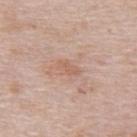<record>
<patient>
  <sex>female</sex>
  <age_approx>65</age_approx>
</patient>
<image>
  <source>total-body photography crop</source>
  <field_of_view_mm>15</field_of_view_mm>
</image>
<site>upper back</site>
</record>